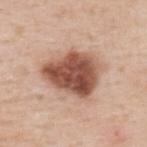<tbp_lesion>
  <biopsy_status>not biopsied; imaged during a skin examination</biopsy_status>
  <site>upper back</site>
  <patient>
    <sex>male</sex>
    <age_approx>50</age_approx>
  </patient>
  <image>
    <source>total-body photography crop</source>
    <field_of_view_mm>15</field_of_view_mm>
  </image>
  <automated_metrics>
    <cielab_L>52</cielab_L>
    <cielab_a>23</cielab_a>
    <cielab_b>29</cielab_b>
    <vs_skin_contrast_norm>12.0</vs_skin_contrast_norm>
  </automated_metrics>
  <lighting>white-light</lighting>
  <lesion_size>
    <long_diameter_mm_approx>6.5</long_diameter_mm_approx>
  </lesion_size>
</tbp_lesion>